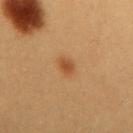<case>
  <biopsy_status>not biopsied; imaged during a skin examination</biopsy_status>
  <lighting>cross-polarized</lighting>
  <image>
    <source>total-body photography crop</source>
    <field_of_view_mm>15</field_of_view_mm>
  </image>
  <site>mid back</site>
  <automated_metrics>
    <vs_skin_darker_L>9.0</vs_skin_darker_L>
    <vs_skin_contrast_norm>7.5</vs_skin_contrast_norm>
    <nevus_likeness_0_100>95</nevus_likeness_0_100>
    <lesion_detection_confidence_0_100>100</lesion_detection_confidence_0_100>
  </automated_metrics>
  <patient>
    <sex>female</sex>
    <age_approx>30</age_approx>
  </patient>
</case>